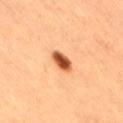Q: Was a biopsy performed?
A: catalogued during a skin exam; not biopsied
Q: What did automated image analysis measure?
A: a lesion area of about 4.5 mm² and a symmetry-axis asymmetry near 0.2; an automated nevus-likeness rating near 100 out of 100
Q: How large is the lesion?
A: about 3 mm
Q: Where on the body is the lesion?
A: the back
Q: What are the patient's age and sex?
A: female, about 45 years old
Q: How was this image acquired?
A: ~15 mm crop, total-body skin-cancer survey